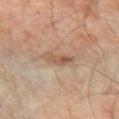Captured during whole-body skin photography for melanoma surveillance; the lesion was not biopsied. A lesion tile, about 15 mm wide, cut from a 3D total-body photograph. The lesion is located on the left forearm. A male subject, aged 43–47.